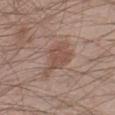{"biopsy_status": "not biopsied; imaged during a skin examination", "automated_metrics": {"area_mm2_approx": 10.0, "cielab_L": 50, "cielab_a": 18, "cielab_b": 25, "vs_skin_darker_L": 8.0, "vs_skin_contrast_norm": 6.0, "nevus_likeness_0_100": 70, "lesion_detection_confidence_0_100": 100}, "lighting": "white-light", "patient": {"sex": "male", "age_approx": 35}, "site": "left lower leg", "image": {"source": "total-body photography crop", "field_of_view_mm": 15}}